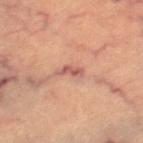Assessment: This lesion was catalogued during total-body skin photography and was not selected for biopsy. Image and clinical context: The total-body-photography lesion software estimated an outline eccentricity of about 0.9 (0 = round, 1 = elongated) and two-axis asymmetry of about 0.35. The software also gave a nevus-likeness score of about 0/100. From the leg. Captured under cross-polarized illumination. A female patient approximately 65 years of age. Cropped from a whole-body photographic skin survey; the tile spans about 15 mm.